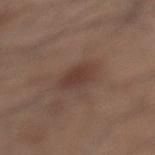The lesion was tiled from a total-body skin photograph and was not biopsied. The subject is a male aged 53–57. This is a white-light tile. An algorithmic analysis of the crop reported an area of roughly 6.5 mm², an outline eccentricity of about 0.25 (0 = round, 1 = elongated), and two-axis asymmetry of about 0.2. And it measured a border-irregularity index near 2/10, internal color variation of about 1.5 on a 0–10 scale, and a peripheral color-asymmetry measure near 0.5. The analysis additionally found an automated nevus-likeness rating near 80 out of 100. Cropped from a total-body skin-imaging series; the visible field is about 15 mm. About 3 mm across. From the left lower leg.